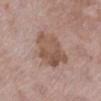– notes: total-body-photography surveillance lesion; no biopsy
– lesion size: ~5.5 mm (longest diameter)
– body site: the left lower leg
– image-analysis metrics: a shape eccentricity near 0.7 and two-axis asymmetry of about 0.25; an average lesion color of about L≈53 a*≈18 b*≈26 (CIELAB), a lesion–skin lightness drop of about 9, and a normalized lesion–skin contrast near 7; internal color variation of about 4 on a 0–10 scale and radial color variation of about 1.5
– imaging modality: ~15 mm crop, total-body skin-cancer survey
– subject: female, aged approximately 70